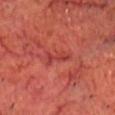<tbp_lesion>
<biopsy_status>not biopsied; imaged during a skin examination</biopsy_status>
<lesion_size>
  <long_diameter_mm_approx>3.0</long_diameter_mm_approx>
</lesion_size>
<site>head or neck</site>
<automated_metrics>
  <border_irregularity_0_10>5.5</border_irregularity_0_10>
  <peripheral_color_asymmetry>0.0</peripheral_color_asymmetry>
</automated_metrics>
<patient>
  <sex>male</sex>
  <age_approx>70</age_approx>
</patient>
<lighting>cross-polarized</lighting>
<image>
  <source>total-body photography crop</source>
  <field_of_view_mm>15</field_of_view_mm>
</image>
</tbp_lesion>